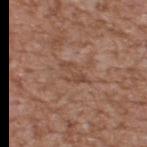The lesion was tiled from a total-body skin photograph and was not biopsied. This is a white-light tile. A close-up tile cropped from a whole-body skin photograph, about 15 mm across. Longest diameter approximately 3 mm. A male patient aged 63 to 67. Located on the upper back. The total-body-photography lesion software estimated border irregularity of about 6 on a 0–10 scale, internal color variation of about 1 on a 0–10 scale, and radial color variation of about 0.5.